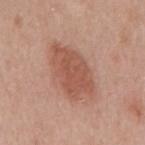Recorded during total-body skin imaging; not selected for excision or biopsy. A female subject, aged approximately 40. Approximately 7 mm at its widest. The lesion is on the back. A 15 mm crop from a total-body photograph taken for skin-cancer surveillance.